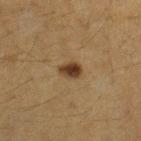Part of a total-body skin-imaging series; this lesion was reviewed on a skin check and was not flagged for biopsy. A female subject, about 55 years old. This image is a 15 mm lesion crop taken from a total-body photograph. From the left forearm.